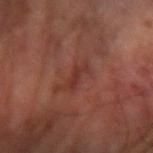workup=total-body-photography surveillance lesion; no biopsy | anatomic site=the right arm | lesion diameter=~3 mm (longest diameter) | subject=male, approximately 60 years of age | tile lighting=cross-polarized illumination | TBP lesion metrics=a mean CIELAB color near L≈32 a*≈25 b*≈25 and a normalized border contrast of about 6; a border-irregularity rating of about 4/10 and peripheral color asymmetry of about 0; an automated nevus-likeness rating near 0 out of 100 and a lesion-detection confidence of about 80/100 | image source=15 mm crop, total-body photography.